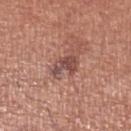Q: Was this lesion biopsied?
A: catalogued during a skin exam; not biopsied
Q: Where on the body is the lesion?
A: the leg
Q: What are the patient's age and sex?
A: male, in their 70s
Q: Illumination type?
A: white-light
Q: What is the imaging modality?
A: ~15 mm tile from a whole-body skin photo